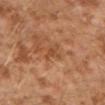<tbp_lesion>
  <biopsy_status>not biopsied; imaged during a skin examination</biopsy_status>
  <automated_metrics>
    <area_mm2_approx>3.0</area_mm2_approx>
    <eccentricity>0.9</eccentricity>
    <shape_asymmetry>0.3</shape_asymmetry>
    <border_irregularity_0_10>4.0</border_irregularity_0_10>
    <color_variation_0_10>0.5</color_variation_0_10>
    <peripheral_color_asymmetry>0.0</peripheral_color_asymmetry>
  </automated_metrics>
  <site>left lower leg</site>
  <patient>
    <sex>male</sex>
    <age_approx>30</age_approx>
  </patient>
  <lighting>cross-polarized</lighting>
  <lesion_size>
    <long_diameter_mm_approx>3.0</long_diameter_mm_approx>
  </lesion_size>
  <image>
    <source>total-body photography crop</source>
    <field_of_view_mm>15</field_of_view_mm>
  </image>
</tbp_lesion>